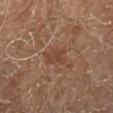The total-body-photography lesion software estimated a border-irregularity index near 4/10, a within-lesion color-variation index near 1.5/10, and a peripheral color-asymmetry measure near 0.5. The software also gave a classifier nevus-likeness of about 0/100 and a detector confidence of about 100 out of 100 that the crop contains a lesion.
The lesion's longest dimension is about 3.5 mm.
The patient is a male about 70 years old.
The lesion is located on the right lower leg.
Captured under cross-polarized illumination.
A roughly 15 mm field-of-view crop from a total-body skin photograph.